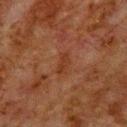A male subject, aged 78–82. A region of skin cropped from a whole-body photographic capture, roughly 15 mm wide. On the upper back.